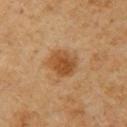The lesion was photographed on a routine skin check and not biopsied; there is no pathology result.